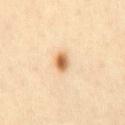Image and clinical context: Automated image analysis of the tile measured radial color variation of about 1.5. The patient is a male about 30 years old. Located on the back. A 15 mm close-up extracted from a 3D total-body photography capture.